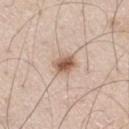Recorded during total-body skin imaging; not selected for excision or biopsy.
Longest diameter approximately 3 mm.
An algorithmic analysis of the crop reported a shape eccentricity near 0.65 and two-axis asymmetry of about 0.2. The analysis additionally found roughly 14 lightness units darker than nearby skin and a normalized lesion–skin contrast near 9.
A region of skin cropped from a whole-body photographic capture, roughly 15 mm wide.
A male subject, in their 60s.
Located on the right thigh.
Captured under white-light illumination.